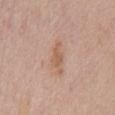subject = male, aged approximately 60
body site = the chest
diameter = about 4 mm
image = 15 mm crop, total-body photography